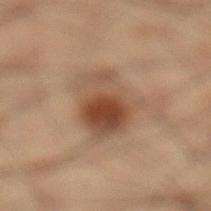The lesion was photographed on a routine skin check and not biopsied; there is no pathology result.
A roughly 15 mm field-of-view crop from a total-body skin photograph.
Automated image analysis of the tile measured an outline eccentricity of about 0.8 (0 = round, 1 = elongated) and a symmetry-axis asymmetry near 0.25. And it measured a mean CIELAB color near L≈36 a*≈15 b*≈24, a lesion–skin lightness drop of about 9, and a normalized border contrast of about 8.5. It also reported a border-irregularity index near 3.5/10, a within-lesion color-variation index near 8/10, and peripheral color asymmetry of about 2.5. The analysis additionally found an automated nevus-likeness rating near 95 out of 100.
Captured under cross-polarized illumination.
The lesion's longest dimension is about 6 mm.
On the leg.
The patient is a male aged 48 to 52.